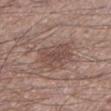Imaged during a routine full-body skin examination; the lesion was not biopsied and no histopathology is available.
A male patient in their mid- to late 50s.
From the left lower leg.
The tile uses white-light illumination.
A lesion tile, about 15 mm wide, cut from a 3D total-body photograph.
The lesion's longest dimension is about 4 mm.